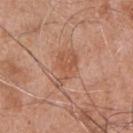biopsy_status: not biopsied; imaged during a skin examination
patient:
  sex: male
  age_approx: 55
site: chest
lesion_size:
  long_diameter_mm_approx: 4.5
automated_metrics:
  cielab_L: 54
  cielab_a: 23
  cielab_b: 32
  vs_skin_darker_L: 7.0
  vs_skin_contrast_norm: 5.5
  color_variation_0_10: 2.0
  peripheral_color_asymmetry: 0.5
lighting: white-light
image:
  source: total-body photography crop
  field_of_view_mm: 15